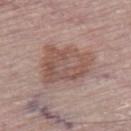| field | value |
|---|---|
| follow-up | catalogued during a skin exam; not biopsied |